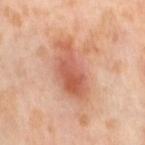{
  "biopsy_status": "not biopsied; imaged during a skin examination",
  "image": {
    "source": "total-body photography crop",
    "field_of_view_mm": 15
  },
  "automated_metrics": {
    "vs_skin_darker_L": 11.0,
    "border_irregularity_0_10": 6.5,
    "color_variation_0_10": 7.0,
    "peripheral_color_asymmetry": 2.0,
    "nevus_likeness_0_100": 60,
    "lesion_detection_confidence_0_100": 100
  },
  "patient": {
    "sex": "female",
    "age_approx": 55
  },
  "site": "leg"
}